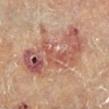<record>
<biopsy_status>not biopsied; imaged during a skin examination</biopsy_status>
<lighting>cross-polarized</lighting>
<patient>
  <sex>male</sex>
  <age_approx>85+</age_approx>
</patient>
<site>right lower leg</site>
<image>
  <source>total-body photography crop</source>
  <field_of_view_mm>15</field_of_view_mm>
</image>
</record>